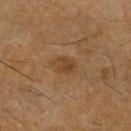Case summary:
– workup — catalogued during a skin exam; not biopsied
– size — ~3 mm (longest diameter)
– image-analysis metrics — a footprint of about 4.5 mm², an outline eccentricity of about 0.7 (0 = round, 1 = elongated), and a symmetry-axis asymmetry near 0.15; a lesion color around L≈40 a*≈18 b*≈33 in CIELAB and a normalized border contrast of about 6.5; a border-irregularity index near 2/10, internal color variation of about 2 on a 0–10 scale, and a peripheral color-asymmetry measure near 1
– imaging modality — ~15 mm crop, total-body skin-cancer survey
– illumination — cross-polarized illumination
– patient — male, approximately 60 years of age
– body site — the leg